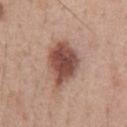Imaged during a routine full-body skin examination; the lesion was not biopsied and no histopathology is available. A 15 mm close-up tile from a total-body photography series done for melanoma screening. Measured at roughly 6.5 mm in maximum diameter. A male patient approximately 55 years of age. Captured under white-light illumination. On the chest.